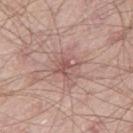Q: Was this lesion biopsied?
A: no biopsy performed (imaged during a skin exam)
Q: What lighting was used for the tile?
A: white-light
Q: Patient demographics?
A: male, roughly 65 years of age
Q: What is the imaging modality?
A: ~15 mm tile from a whole-body skin photo
Q: What is the anatomic site?
A: the right thigh
Q: What is the lesion's diameter?
A: about 3 mm